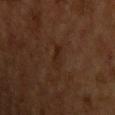No biopsy was performed on this lesion — it was imaged during a full skin examination and was not determined to be concerning. On the chest. This image is a 15 mm lesion crop taken from a total-body photograph. A male patient, aged around 60. Automated tile analysis of the lesion measured an area of roughly 3 mm² and a shape-asymmetry score of about 0.25 (0 = symmetric). The analysis additionally found lesion-presence confidence of about 95/100. About 3 mm across.